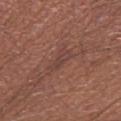Clinical impression:
Imaged during a routine full-body skin examination; the lesion was not biopsied and no histopathology is available.
Clinical summary:
The lesion's longest dimension is about 3 mm. A 15 mm crop from a total-body photograph taken for skin-cancer surveillance. The lesion is located on the right thigh. The lesion-visualizer software estimated a mean CIELAB color near L≈41 a*≈20 b*≈23, about 5 CIELAB-L* units darker than the surrounding skin, and a normalized border contrast of about 5. It also reported a nevus-likeness score of about 0/100 and a detector confidence of about 80 out of 100 that the crop contains a lesion. Imaged with white-light lighting. The subject is a male aged approximately 45.